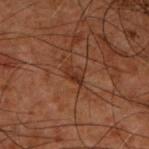Captured during whole-body skin photography for melanoma surveillance; the lesion was not biopsied.
The lesion is located on the back.
The subject is a male approximately 50 years of age.
A lesion tile, about 15 mm wide, cut from a 3D total-body photograph.